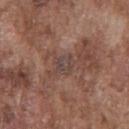This lesion was catalogued during total-body skin photography and was not selected for biopsy.